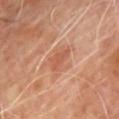biopsy status: no biopsy performed (imaged during a skin exam)
location: the chest
lesion diameter: about 3.5 mm
lighting: cross-polarized
subject: male, roughly 65 years of age
image source: total-body-photography crop, ~15 mm field of view
TBP lesion metrics: a mean CIELAB color near L≈53 a*≈25 b*≈32 and a normalized border contrast of about 5.5; border irregularity of about 4 on a 0–10 scale and peripheral color asymmetry of about 0.5